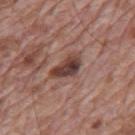• workup: catalogued during a skin exam; not biopsied
• acquisition: 15 mm crop, total-body photography
• anatomic site: the mid back
• patient: male, approximately 70 years of age